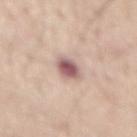Impression:
The lesion was tiled from a total-body skin photograph and was not biopsied.
Acquisition and patient details:
Cropped from a total-body skin-imaging series; the visible field is about 15 mm. The recorded lesion diameter is about 3 mm. On the mid back. Captured under white-light illumination. A male patient, approximately 55 years of age.